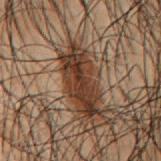| field | value |
|---|---|
| follow-up | total-body-photography surveillance lesion; no biopsy |
| subject | male, approximately 50 years of age |
| size | ≈7 mm |
| image | total-body-photography crop, ~15 mm field of view |
| illumination | cross-polarized illumination |
| anatomic site | the mid back |